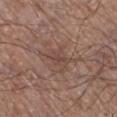Clinical impression:
The lesion was photographed on a routine skin check and not biopsied; there is no pathology result.
Acquisition and patient details:
A male subject, aged 63–67. A close-up tile cropped from a whole-body skin photograph, about 15 mm across. From the right lower leg. About 3 mm across.